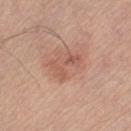Assessment: Imaged during a routine full-body skin examination; the lesion was not biopsied and no histopathology is available. Image and clinical context: A female patient, aged 63 to 67. Measured at roughly 4 mm in maximum diameter. Automated image analysis of the tile measured a lesion area of about 6.5 mm², an outline eccentricity of about 0.8 (0 = round, 1 = elongated), and two-axis asymmetry of about 0.45. The analysis additionally found an average lesion color of about L≈56 a*≈23 b*≈29 (CIELAB) and roughly 8 lightness units darker than nearby skin. And it measured internal color variation of about 4 on a 0–10 scale. And it measured an automated nevus-likeness rating near 5 out of 100. Imaged with white-light lighting. Located on the right thigh. A lesion tile, about 15 mm wide, cut from a 3D total-body photograph.